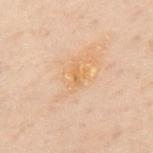Findings:
* workup: no biopsy performed (imaged during a skin exam)
* body site: the mid back
* diameter: about 2.5 mm
* automated lesion analysis: a lesion color around L≈55 a*≈17 b*≈34 in CIELAB and a lesion–skin lightness drop of about 5; a nevus-likeness score of about 0/100 and lesion-presence confidence of about 100/100
* subject: male, aged 48–52
* tile lighting: cross-polarized illumination
* image: ~15 mm tile from a whole-body skin photo